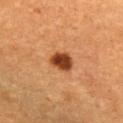Q: Was a biopsy performed?
A: imaged on a skin check; not biopsied
Q: How was this image acquired?
A: ~15 mm crop, total-body skin-cancer survey
Q: Automated lesion metrics?
A: an automated nevus-likeness rating near 100 out of 100 and lesion-presence confidence of about 100/100
Q: Who is the patient?
A: female, in their mid-50s
Q: What is the lesion's diameter?
A: about 3 mm
Q: Where on the body is the lesion?
A: the chest